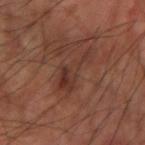Recorded during total-body skin imaging; not selected for excision or biopsy. A male subject, approximately 60 years of age. The recorded lesion diameter is about 6 mm. A 15 mm close-up tile from a total-body photography series done for melanoma screening. An algorithmic analysis of the crop reported an area of roughly 9.5 mm², a shape eccentricity near 0.9, and two-axis asymmetry of about 0.65. It also reported a nevus-likeness score of about 10/100 and lesion-presence confidence of about 100/100. This is a cross-polarized tile. Located on the left arm.